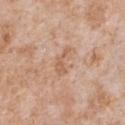Assessment: Imaged during a routine full-body skin examination; the lesion was not biopsied and no histopathology is available. Background: A 15 mm crop from a total-body photograph taken for skin-cancer surveillance. A male subject, aged approximately 65. Measured at roughly 3.5 mm in maximum diameter. From the chest. An algorithmic analysis of the crop reported an average lesion color of about L≈60 a*≈20 b*≈33 (CIELAB) and about 8 CIELAB-L* units darker than the surrounding skin. The analysis additionally found a nevus-likeness score of about 0/100 and a lesion-detection confidence of about 100/100.